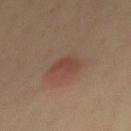Impression:
The lesion was tiled from a total-body skin photograph and was not biopsied.
Acquisition and patient details:
The lesion's longest dimension is about 3 mm. From the chest. A male patient, roughly 40 years of age. Automated tile analysis of the lesion measured an area of roughly 4.5 mm², a shape eccentricity near 0.7, and a symmetry-axis asymmetry near 0.45. The software also gave a border-irregularity index near 4/10 and a peripheral color-asymmetry measure near 0.5. The software also gave an automated nevus-likeness rating near 100 out of 100 and a lesion-detection confidence of about 100/100. This image is a 15 mm lesion crop taken from a total-body photograph. Captured under cross-polarized illumination.